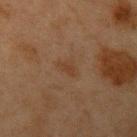Q: Was a biopsy performed?
A: no biopsy performed (imaged during a skin exam)
Q: Lesion location?
A: the left upper arm
Q: How large is the lesion?
A: about 3 mm
Q: What lighting was used for the tile?
A: cross-polarized illumination
Q: Who is the patient?
A: female, in their 40s
Q: What did automated image analysis measure?
A: an area of roughly 2.5 mm², a shape eccentricity near 0.9, and a shape-asymmetry score of about 0.4 (0 = symmetric)
Q: What is the imaging modality?
A: ~15 mm tile from a whole-body skin photo